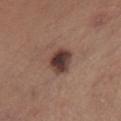Notes:
* biopsy status: no biopsy performed (imaged during a skin exam)
* image source: ~15 mm crop, total-body skin-cancer survey
* patient: female, in their mid- to late 50s
* lighting: white-light
* size: ≈3.5 mm
* image-analysis metrics: a footprint of about 8 mm², a shape eccentricity near 0.5, and two-axis asymmetry of about 0.2
* body site: the chest